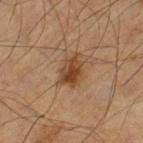{"biopsy_status": "not biopsied; imaged during a skin examination", "lighting": "cross-polarized", "patient": {"sex": "male", "age_approx": 60}, "lesion_size": {"long_diameter_mm_approx": 3.5}, "automated_metrics": {"eccentricity": 0.75, "cielab_L": 39, "cielab_a": 18, "cielab_b": 31, "vs_skin_darker_L": 10.0, "vs_skin_contrast_norm": 9.0, "nevus_likeness_0_100": 90}, "image": {"source": "total-body photography crop", "field_of_view_mm": 15}, "site": "leg"}